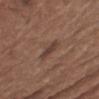The lesion was tiled from a total-body skin photograph and was not biopsied.
From the left upper arm.
The recorded lesion diameter is about 2.5 mm.
Captured under white-light illumination.
The patient is a male aged 73–77.
A region of skin cropped from a whole-body photographic capture, roughly 15 mm wide.